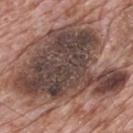<tbp_lesion>
<biopsy_status>not biopsied; imaged during a skin examination</biopsy_status>
<lesion_size>
  <long_diameter_mm_approx>14.5</long_diameter_mm_approx>
</lesion_size>
<automated_metrics>
  <cielab_L>43</cielab_L>
  <cielab_a>17</cielab_a>
  <cielab_b>21</cielab_b>
  <vs_skin_contrast_norm>11.5</vs_skin_contrast_norm>
  <border_irregularity_0_10>6.5</border_irregularity_0_10>
  <peripheral_color_asymmetry>3.0</peripheral_color_asymmetry>
</automated_metrics>
<patient>
  <sex>male</sex>
  <age_approx>70</age_approx>
</patient>
<image>
  <source>total-body photography crop</source>
  <field_of_view_mm>15</field_of_view_mm>
</image>
<site>mid back</site>
<lighting>white-light</lighting>
</tbp_lesion>